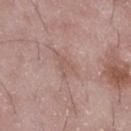Case summary:
– follow-up: imaged on a skin check; not biopsied
– image: ~15 mm crop, total-body skin-cancer survey
– site: the right thigh
– patient: male, about 50 years old